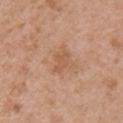Case summary:
– workup: catalogued during a skin exam; not biopsied
– diameter: about 3 mm
– tile lighting: white-light illumination
– acquisition: ~15 mm tile from a whole-body skin photo
– patient: female, in their 40s
– location: the right upper arm
– image-analysis metrics: a nevus-likeness score of about 0/100 and a detector confidence of about 100 out of 100 that the crop contains a lesion